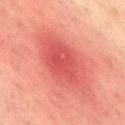Recorded during total-body skin imaging; not selected for excision or biopsy. A 15 mm close-up extracted from a 3D total-body photography capture. The lesion is located on the right thigh. A male patient, about 35 years old.